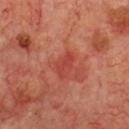Captured during whole-body skin photography for melanoma surveillance; the lesion was not biopsied. A male subject, aged around 70. A 15 mm crop from a total-body photograph taken for skin-cancer surveillance. About 3 mm across. The lesion is located on the chest. The tile uses cross-polarized illumination. Automated tile analysis of the lesion measured roughly 7 lightness units darker than nearby skin and a normalized border contrast of about 5.5. The software also gave a border-irregularity rating of about 5/10 and a peripheral color-asymmetry measure near 0.